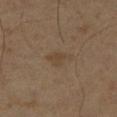Assessment: This lesion was catalogued during total-body skin photography and was not selected for biopsy. Clinical summary: Captured under cross-polarized illumination. This image is a 15 mm lesion crop taken from a total-body photograph. A male patient, aged around 65. Approximately 2.5 mm at its widest.